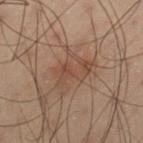notes = total-body-photography surveillance lesion; no biopsy
patient = male, in their mid-50s
location = the left thigh
image source = ~15 mm tile from a whole-body skin photo